A 15 mm close-up tile from a total-body photography series done for melanoma screening. From the leg. A male patient, in their mid- to late 50s.
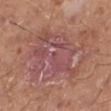Measured at roughly 8.5 mm in maximum diameter. This is a white-light tile. Histopathological examination showed a superficial basal cell carcinoma (malignant).